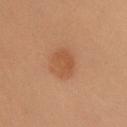Clinical impression:
The lesion was photographed on a routine skin check and not biopsied; there is no pathology result.
Clinical summary:
From the front of the torso. Measured at roughly 3 mm in maximum diameter. This image is a 15 mm lesion crop taken from a total-body photograph. This is a cross-polarized tile. A female subject aged 38–42.